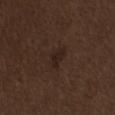No biopsy was performed on this lesion — it was imaged during a full skin examination and was not determined to be concerning. Imaged with white-light lighting. A 15 mm close-up tile from a total-body photography series done for melanoma screening. The lesion's longest dimension is about 3 mm. Located on the chest. A male patient aged 68 to 72.